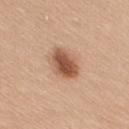Recorded during total-body skin imaging; not selected for excision or biopsy. The total-body-photography lesion software estimated an area of roughly 8.5 mm² and an eccentricity of roughly 0.75. And it measured a nevus-likeness score of about 95/100. Longest diameter approximately 4 mm. The patient is a female roughly 20 years of age. Captured under white-light illumination. The lesion is on the arm. Cropped from a whole-body photographic skin survey; the tile spans about 15 mm.